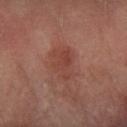Case summary:
– biopsy status — catalogued during a skin exam; not biopsied
– image — 15 mm crop, total-body photography
– site — the right forearm
– subject — male, about 65 years old
– size — ≈4 mm